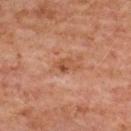Q: Is there a histopathology result?
A: catalogued during a skin exam; not biopsied
Q: Who is the patient?
A: female, roughly 60 years of age
Q: Where on the body is the lesion?
A: the upper back
Q: What did automated image analysis measure?
A: a lesion area of about 3 mm² and a symmetry-axis asymmetry near 0.35; a lesion color around L≈45 a*≈22 b*≈31 in CIELAB, about 8 CIELAB-L* units darker than the surrounding skin, and a normalized border contrast of about 6.5
Q: How large is the lesion?
A: about 2.5 mm
Q: How was this image acquired?
A: total-body-photography crop, ~15 mm field of view